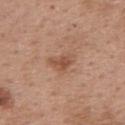follow-up = total-body-photography surveillance lesion; no biopsy
subject = female, approximately 40 years of age
diameter = about 3.5 mm
body site = the upper back
image source = 15 mm crop, total-body photography
TBP lesion metrics = a lesion area of about 5 mm², a shape eccentricity near 0.8, and a symmetry-axis asymmetry near 0.4